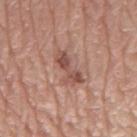* diameter — ≈4.5 mm
* image — 15 mm crop, total-body photography
* lighting — white-light illumination
* anatomic site — the back
* automated metrics — an outline eccentricity of about 0.9 (0 = round, 1 = elongated) and two-axis asymmetry of about 0.3; an average lesion color of about L≈51 a*≈22 b*≈25 (CIELAB), roughly 10 lightness units darker than nearby skin, and a lesion-to-skin contrast of about 7.5 (normalized; higher = more distinct); a border-irregularity rating of about 4.5/10 and peripheral color asymmetry of about 1.5; a classifier nevus-likeness of about 10/100 and lesion-presence confidence of about 100/100
* patient — male, roughly 80 years of age